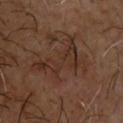{
  "biopsy_status": "not biopsied; imaged during a skin examination",
  "patient": {
    "sex": "male",
    "age_approx": 65
  },
  "lighting": "cross-polarized",
  "lesion_size": {
    "long_diameter_mm_approx": 6.5
  },
  "image": {
    "source": "total-body photography crop",
    "field_of_view_mm": 15
  },
  "site": "upper back"
}